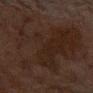Captured during whole-body skin photography for melanoma surveillance; the lesion was not biopsied.
A region of skin cropped from a whole-body photographic capture, roughly 15 mm wide.
The recorded lesion diameter is about 13.5 mm.
This is a cross-polarized tile.
A female subject aged 48 to 52.
Automated image analysis of the tile measured a lesion color around L≈18 a*≈12 b*≈17 in CIELAB, roughly 4 lightness units darker than nearby skin, and a lesion-to-skin contrast of about 5.5 (normalized; higher = more distinct). The analysis additionally found a border-irregularity rating of about 7.5/10 and internal color variation of about 4 on a 0–10 scale.
The lesion is located on the arm.